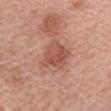Part of a total-body skin-imaging series; this lesion was reviewed on a skin check and was not flagged for biopsy.
Imaged with white-light lighting.
The lesion's longest dimension is about 3.5 mm.
The lesion is on the arm.
A female subject, aged 63 to 67.
This image is a 15 mm lesion crop taken from a total-body photograph.